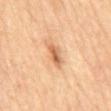Notes:
– biopsy status · catalogued during a skin exam; not biopsied
– image-analysis metrics · a footprint of about 5 mm² and a shape eccentricity near 0.9; a mean CIELAB color near L≈63 a*≈23 b*≈38, a lesion–skin lightness drop of about 12, and a normalized border contrast of about 7.5; a nevus-likeness score of about 60/100 and a detector confidence of about 100 out of 100 that the crop contains a lesion
– lighting · cross-polarized
– acquisition · ~15 mm crop, total-body skin-cancer survey
– location · the mid back
– patient · male, aged approximately 85 or older
– diameter · ≈3.5 mm